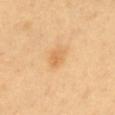Part of a total-body skin-imaging series; this lesion was reviewed on a skin check and was not flagged for biopsy.
The lesion is on the front of the torso.
Approximately 2.5 mm at its widest.
Captured under cross-polarized illumination.
A female subject in their 60s.
A 15 mm close-up extracted from a 3D total-body photography capture.